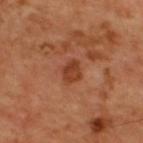Clinical impression: Part of a total-body skin-imaging series; this lesion was reviewed on a skin check and was not flagged for biopsy. Context: The tile uses cross-polarized illumination. The lesion's longest dimension is about 2.5 mm. A region of skin cropped from a whole-body photographic capture, roughly 15 mm wide. The lesion is on the upper back. An algorithmic analysis of the crop reported an area of roughly 4.5 mm², a shape eccentricity near 0.65, and a symmetry-axis asymmetry near 0.2. And it measured a classifier nevus-likeness of about 25/100 and a detector confidence of about 100 out of 100 that the crop contains a lesion. The patient is a male aged 58–62.